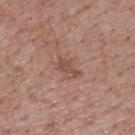workup: total-body-photography surveillance lesion; no biopsy | automated metrics: about 8 CIELAB-L* units darker than the surrounding skin and a lesion-to-skin contrast of about 6 (normalized; higher = more distinct) | tile lighting: white-light illumination | image source: 15 mm crop, total-body photography | lesion size: ~3 mm (longest diameter) | body site: the upper back | patient: male, in their 70s.